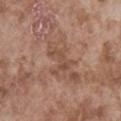<lesion>
<biopsy_status>not biopsied; imaged during a skin examination</biopsy_status>
<lesion_size>
  <long_diameter_mm_approx>3.5</long_diameter_mm_approx>
</lesion_size>
<lighting>white-light</lighting>
<site>abdomen</site>
<patient>
  <sex>male</sex>
  <age_approx>75</age_approx>
</patient>
<image>
  <source>total-body photography crop</source>
  <field_of_view_mm>15</field_of_view_mm>
</image>
</lesion>